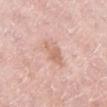| field | value |
|---|---|
| follow-up | catalogued during a skin exam; not biopsied |
| acquisition | total-body-photography crop, ~15 mm field of view |
| lighting | white-light illumination |
| site | the right lower leg |
| patient | female, in their 70s |